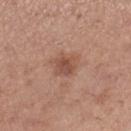This lesion was catalogued during total-body skin photography and was not selected for biopsy. A close-up tile cropped from a whole-body skin photograph, about 15 mm across. The lesion is on the right lower leg. The lesion's longest dimension is about 3 mm. The lesion-visualizer software estimated a lesion area of about 5 mm², an eccentricity of roughly 0.5, and two-axis asymmetry of about 0.25. It also reported border irregularity of about 2.5 on a 0–10 scale, a within-lesion color-variation index near 3/10, and a peripheral color-asymmetry measure near 1. It also reported an automated nevus-likeness rating near 25 out of 100. Captured under white-light illumination. A female subject, roughly 30 years of age.